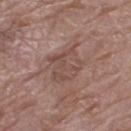{"biopsy_status": "not biopsied; imaged during a skin examination", "lesion_size": {"long_diameter_mm_approx": 4.5}, "image": {"source": "total-body photography crop", "field_of_view_mm": 15}, "lighting": "white-light", "patient": {"sex": "female", "age_approx": 70}, "site": "left thigh"}